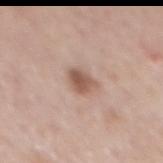  biopsy_status: not biopsied; imaged during a skin examination
  patient:
    sex: female
    age_approx: 65
  site: back
  image:
    source: total-body photography crop
    field_of_view_mm: 15